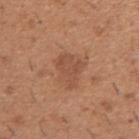biopsy_status: not biopsied; imaged during a skin examination
lighting: white-light
image:
  source: total-body photography crop
  field_of_view_mm: 15
site: right upper arm
lesion_size:
  long_diameter_mm_approx: 4.0
patient:
  sex: male
  age_approx: 40